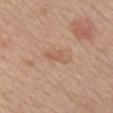| field | value |
|---|---|
| workup | no biopsy performed (imaged during a skin exam) |
| image source | total-body-photography crop, ~15 mm field of view |
| lighting | white-light |
| size | ≈3 mm |
| body site | the chest |
| subject | female, aged 48–52 |